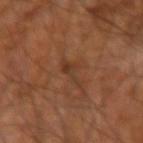{"biopsy_status": "not biopsied; imaged during a skin examination", "site": "right forearm", "lesion_size": {"long_diameter_mm_approx": 4.0}, "patient": {"sex": "male", "age_approx": 60}, "lighting": "cross-polarized", "image": {"source": "total-body photography crop", "field_of_view_mm": 15}, "automated_metrics": {"area_mm2_approx": 3.5, "eccentricity": 0.9, "shape_asymmetry": 0.5, "cielab_L": 35, "cielab_a": 20, "cielab_b": 29, "vs_skin_darker_L": 6.0, "vs_skin_contrast_norm": 6.0, "border_irregularity_0_10": 6.5, "color_variation_0_10": 1.5, "peripheral_color_asymmetry": 0.0, "lesion_detection_confidence_0_100": 95}}